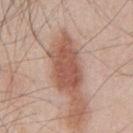Clinical impression:
The lesion was tiled from a total-body skin photograph and was not biopsied.
Context:
A male subject in their mid- to late 40s. Approximately 6.5 mm at its widest. Cropped from a total-body skin-imaging series; the visible field is about 15 mm. The lesion is on the chest. The tile uses white-light illumination. The total-body-photography lesion software estimated an area of roughly 16 mm² and a shape eccentricity near 0.85. And it measured a mean CIELAB color near L≈54 a*≈23 b*≈28 and about 13 CIELAB-L* units darker than the surrounding skin. And it measured a lesion-detection confidence of about 100/100.